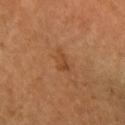follow-up: total-body-photography surveillance lesion; no biopsy | acquisition: total-body-photography crop, ~15 mm field of view | site: the right forearm | patient: female, in their 60s | size: ~3 mm (longest diameter) | tile lighting: cross-polarized illumination.